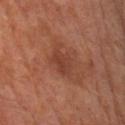Part of a total-body skin-imaging series; this lesion was reviewed on a skin check and was not flagged for biopsy. A male patient, aged 63–67. A lesion tile, about 15 mm wide, cut from a 3D total-body photograph. The tile uses cross-polarized illumination. The lesion is on the right upper arm. Longest diameter approximately 3 mm.